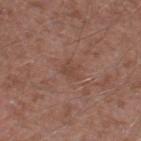Imaged during a routine full-body skin examination; the lesion was not biopsied and no histopathology is available. Imaged with white-light lighting. The lesion's longest dimension is about 2.5 mm. A male subject, aged 58 to 62. Automated image analysis of the tile measured an area of roughly 3.5 mm² and a shape eccentricity near 0.75. And it measured roughly 5 lightness units darker than nearby skin and a lesion-to-skin contrast of about 4.5 (normalized; higher = more distinct). It also reported border irregularity of about 4 on a 0–10 scale, a color-variation rating of about 1/10, and radial color variation of about 0.5. The analysis additionally found a detector confidence of about 100 out of 100 that the crop contains a lesion. A region of skin cropped from a whole-body photographic capture, roughly 15 mm wide. The lesion is located on the left thigh.